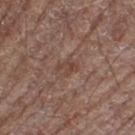This lesion was catalogued during total-body skin photography and was not selected for biopsy. The tile uses white-light illumination. The subject is a male aged approximately 70. The recorded lesion diameter is about 3 mm. Located on the left thigh. Cropped from a whole-body photographic skin survey; the tile spans about 15 mm.